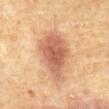Assessment:
The lesion was tiled from a total-body skin photograph and was not biopsied.